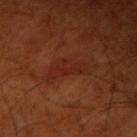workup = imaged on a skin check; not biopsied
diameter = about 5 mm
image source = ~15 mm tile from a whole-body skin photo
patient = male, roughly 70 years of age
illumination = cross-polarized illumination
anatomic site = the right upper arm